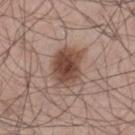– lighting · white-light
– image source · 15 mm crop, total-body photography
– patient · male, aged around 70
– diameter · about 4.5 mm
– site · the lower back